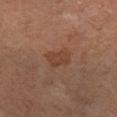follow-up = catalogued during a skin exam; not biopsied.